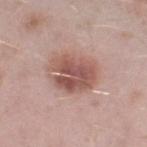Case summary:
* biopsy status — imaged on a skin check; not biopsied
* lesion size — about 5.5 mm
* anatomic site — the right lower leg
* lighting — white-light illumination
* patient — male, aged 38–42
* image — total-body-photography crop, ~15 mm field of view